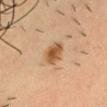Impression:
This lesion was catalogued during total-body skin photography and was not selected for biopsy.
Background:
On the chest. A male patient in their mid- to late 30s. The recorded lesion diameter is about 3 mm. Automated image analysis of the tile measured an area of roughly 6.5 mm², an outline eccentricity of about 0.6 (0 = round, 1 = elongated), and two-axis asymmetry of about 0.2. It also reported an average lesion color of about L≈49 a*≈18 b*≈34 (CIELAB) and a normalized border contrast of about 9. The software also gave a classifier nevus-likeness of about 95/100. Cropped from a total-body skin-imaging series; the visible field is about 15 mm.